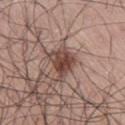Impression: Captured during whole-body skin photography for melanoma surveillance; the lesion was not biopsied. Clinical summary: This image is a 15 mm lesion crop taken from a total-body photograph. The lesion's longest dimension is about 4 mm. From the lower back. An algorithmic analysis of the crop reported a lesion area of about 9 mm². It also reported about 13 CIELAB-L* units darker than the surrounding skin and a normalized lesion–skin contrast near 9.5. The analysis additionally found a border-irregularity index near 4/10, a color-variation rating of about 4.5/10, and radial color variation of about 1.5. A male subject aged approximately 70.